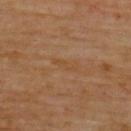notes = total-body-photography surveillance lesion; no biopsy | anatomic site = the upper back | tile lighting = cross-polarized | diameter = ≈3 mm | patient = male, about 60 years old | imaging modality = total-body-photography crop, ~15 mm field of view.